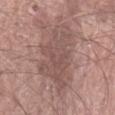Imaged during a routine full-body skin examination; the lesion was not biopsied and no histopathology is available. Longest diameter approximately 8.5 mm. The total-body-photography lesion software estimated a footprint of about 27 mm² and a symmetry-axis asymmetry near 0.4. The analysis additionally found a mean CIELAB color near L≈51 a*≈18 b*≈21 and a normalized lesion–skin contrast near 6.5. The software also gave a within-lesion color-variation index near 3/10 and a peripheral color-asymmetry measure near 1. The software also gave an automated nevus-likeness rating near 0 out of 100. The patient is a male aged 58 to 62. A lesion tile, about 15 mm wide, cut from a 3D total-body photograph. On the left forearm. Captured under white-light illumination.